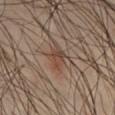No biopsy was performed on this lesion — it was imaged during a full skin examination and was not determined to be concerning.
Automated tile analysis of the lesion measured an area of roughly 5.5 mm², a shape eccentricity near 0.8, and a shape-asymmetry score of about 0.25 (0 = symmetric). The software also gave a lesion–skin lightness drop of about 7 and a normalized border contrast of about 6. And it measured a color-variation rating of about 3.5/10 and peripheral color asymmetry of about 1. The analysis additionally found an automated nevus-likeness rating near 85 out of 100.
About 3.5 mm across.
Captured under cross-polarized illumination.
A male patient approximately 40 years of age.
A 15 mm close-up tile from a total-body photography series done for melanoma screening.
Located on the abdomen.